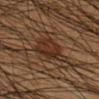Clinical summary: The lesion-visualizer software estimated an outline eccentricity of about 0.8 (0 = round, 1 = elongated) and two-axis asymmetry of about 0.35. A male subject aged approximately 45. The tile uses cross-polarized illumination. A close-up tile cropped from a whole-body skin photograph, about 15 mm across. On the right lower leg. Longest diameter approximately 5.5 mm.